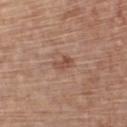- anatomic site · the chest
- lighting · white-light illumination
- size · ≈2.5 mm
- patient · male, roughly 70 years of age
- imaging modality · ~15 mm tile from a whole-body skin photo
- automated metrics · peripheral color asymmetry of about 0.5; an automated nevus-likeness rating near 5 out of 100 and a detector confidence of about 100 out of 100 that the crop contains a lesion